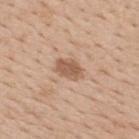A male patient about 60 years old.
Measured at roughly 3 mm in maximum diameter.
The lesion is located on the upper back.
A 15 mm crop from a total-body photograph taken for skin-cancer surveillance.
Captured under white-light illumination.
An algorithmic analysis of the crop reported a lesion color around L≈57 a*≈19 b*≈31 in CIELAB and a normalized border contrast of about 7.5. The analysis additionally found border irregularity of about 2 on a 0–10 scale and a color-variation rating of about 2/10.